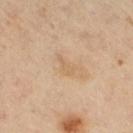Part of a total-body skin-imaging series; this lesion was reviewed on a skin check and was not flagged for biopsy. Cropped from a whole-body photographic skin survey; the tile spans about 15 mm. From the right thigh. A male patient aged approximately 65.